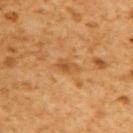follow-up: no biopsy performed (imaged during a skin exam) | size: ≈3 mm | anatomic site: the back | automated metrics: a lesion color around L≈44 a*≈19 b*≈36 in CIELAB and a lesion–skin lightness drop of about 6; border irregularity of about 2.5 on a 0–10 scale; a classifier nevus-likeness of about 0/100 and a lesion-detection confidence of about 100/100 | illumination: cross-polarized illumination | patient: male, aged approximately 60 | image: ~15 mm crop, total-body skin-cancer survey.